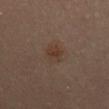{
  "biopsy_status": "not biopsied; imaged during a skin examination",
  "lesion_size": {
    "long_diameter_mm_approx": 3.5
  },
  "site": "mid back",
  "image": {
    "source": "total-body photography crop",
    "field_of_view_mm": 15
  },
  "automated_metrics": {
    "area_mm2_approx": 4.5,
    "eccentricity": 0.8,
    "shape_asymmetry": 0.25,
    "cielab_L": 35,
    "cielab_a": 16,
    "cielab_b": 24,
    "vs_skin_darker_L": 5.0,
    "border_irregularity_0_10": 2.5,
    "peripheral_color_asymmetry": 1.0
  },
  "lighting": "cross-polarized",
  "patient": {
    "sex": "female",
    "age_approx": 45
  }
}